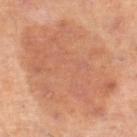Impression: This lesion was catalogued during total-body skin photography and was not selected for biopsy. Image and clinical context: Cropped from a whole-body photographic skin survey; the tile spans about 15 mm. Measured at roughly 12 mm in maximum diameter. Located on the right lower leg. A female subject, aged 38 to 42.